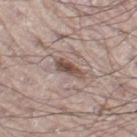Image and clinical context: The tile uses white-light illumination. Approximately 4 mm at its widest. The lesion is on the right leg. The total-body-photography lesion software estimated a footprint of about 4.5 mm², a shape eccentricity near 0.95, and a symmetry-axis asymmetry near 0.35. And it measured a lesion color around L≈49 a*≈16 b*≈22 in CIELAB, a lesion–skin lightness drop of about 13, and a normalized lesion–skin contrast near 9.5. The software also gave a border-irregularity index near 4/10, a within-lesion color-variation index near 2.5/10, and a peripheral color-asymmetry measure near 0.5. The analysis additionally found a nevus-likeness score of about 50/100 and a lesion-detection confidence of about 65/100. A roughly 15 mm field-of-view crop from a total-body skin photograph. The subject is a male about 70 years old.